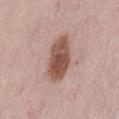Clinical impression:
The lesion was tiled from a total-body skin photograph and was not biopsied.
Background:
The tile uses white-light illumination. Approximately 6 mm at its widest. Located on the lower back. Automated image analysis of the tile measured a lesion-to-skin contrast of about 10.5 (normalized; higher = more distinct). And it measured border irregularity of about 2 on a 0–10 scale and internal color variation of about 4 on a 0–10 scale. And it measured a classifier nevus-likeness of about 100/100 and a detector confidence of about 100 out of 100 that the crop contains a lesion. A female subject, in their 40s. A 15 mm close-up tile from a total-body photography series done for melanoma screening.